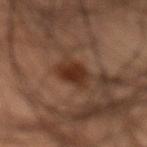The lesion was photographed on a routine skin check and not biopsied; there is no pathology result. The lesion-visualizer software estimated an eccentricity of roughly 0.75. It also reported an automated nevus-likeness rating near 90 out of 100 and a detector confidence of about 100 out of 100 that the crop contains a lesion. The subject is a male roughly 55 years of age. Imaged with cross-polarized lighting. A 15 mm close-up tile from a total-body photography series done for melanoma screening. Longest diameter approximately 4 mm. Located on the front of the torso.